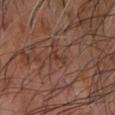Context:
An algorithmic analysis of the crop reported a lesion area of about 2 mm² and a shape eccentricity near 0.95. It also reported an average lesion color of about L≈36 a*≈21 b*≈26 (CIELAB) and roughly 6 lightness units darker than nearby skin. The software also gave border irregularity of about 5 on a 0–10 scale and internal color variation of about 0 on a 0–10 scale. And it measured a nevus-likeness score of about 0/100 and a lesion-detection confidence of about 95/100. From the left forearm. The lesion's longest dimension is about 2.5 mm. A 15 mm close-up extracted from a 3D total-body photography capture. A male patient, aged 63 to 67.